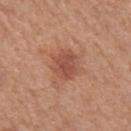biopsy_status: not biopsied; imaged during a skin examination
site: left upper arm
image:
  source: total-body photography crop
  field_of_view_mm: 15
patient:
  sex: female
  age_approx: 50